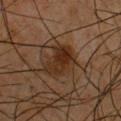<case>
  <site>chest</site>
  <lighting>cross-polarized</lighting>
  <automated_metrics>
    <vs_skin_darker_L>8.0</vs_skin_darker_L>
    <vs_skin_contrast_norm>9.5</vs_skin_contrast_norm>
    <border_irregularity_0_10>4.0</border_irregularity_0_10>
    <color_variation_0_10>4.5</color_variation_0_10>
    <peripheral_color_asymmetry>1.5</peripheral_color_asymmetry>
  </automated_metrics>
  <patient>
    <sex>male</sex>
    <age_approx>65</age_approx>
  </patient>
  <image>
    <source>total-body photography crop</source>
    <field_of_view_mm>15</field_of_view_mm>
  </image>
  <lesion_size>
    <long_diameter_mm_approx>4.5</long_diameter_mm_approx>
  </lesion_size>
</case>